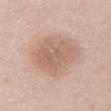biopsy_status: not biopsied; imaged during a skin examination
site: chest
image:
  source: total-body photography crop
  field_of_view_mm: 15
patient:
  sex: female
  age_approx: 60
lighting: white-light